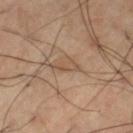biopsy status: imaged on a skin check; not biopsied | automated metrics: an average lesion color of about L≈49 a*≈16 b*≈29 (CIELAB), roughly 7 lightness units darker than nearby skin, and a lesion-to-skin contrast of about 5.5 (normalized; higher = more distinct); a classifier nevus-likeness of about 0/100 and lesion-presence confidence of about 85/100 | acquisition: ~15 mm tile from a whole-body skin photo | size: ~3.5 mm (longest diameter) | patient: male, about 60 years old | tile lighting: cross-polarized | location: the left thigh.